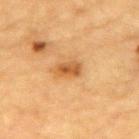Imaged during a routine full-body skin examination; the lesion was not biopsied and no histopathology is available. A 15 mm close-up tile from a total-body photography series done for melanoma screening. From the back. A male subject about 85 years old or older. This is a cross-polarized tile.